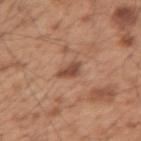This lesion was catalogued during total-body skin photography and was not selected for biopsy. Automated tile analysis of the lesion measured roughly 12 lightness units darker than nearby skin. And it measured a nevus-likeness score of about 25/100 and lesion-presence confidence of about 100/100. The subject is a male approximately 55 years of age. The recorded lesion diameter is about 3 mm. From the right upper arm. A close-up tile cropped from a whole-body skin photograph, about 15 mm across.